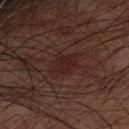<lesion>
  <biopsy_status>not biopsied; imaged during a skin examination</biopsy_status>
  <lesion_size>
    <long_diameter_mm_approx>4.0</long_diameter_mm_approx>
  </lesion_size>
  <automated_metrics>
    <border_irregularity_0_10>2.5</border_irregularity_0_10>
  </automated_metrics>
  <patient>
    <sex>male</sex>
    <age_approx>75</age_approx>
  </patient>
  <lighting>cross-polarized</lighting>
  <site>left forearm</site>
  <image>
    <source>total-body photography crop</source>
    <field_of_view_mm>15</field_of_view_mm>
  </image>
</lesion>